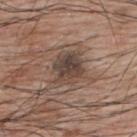Impression: No biopsy was performed on this lesion — it was imaged during a full skin examination and was not determined to be concerning. Context: The lesion is located on the upper back. A 15 mm crop from a total-body photograph taken for skin-cancer surveillance. About 4 mm across. The tile uses white-light illumination. A male patient roughly 70 years of age.